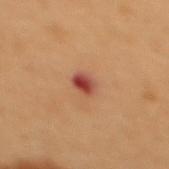  biopsy_status: not biopsied; imaged during a skin examination
  image:
    source: total-body photography crop
    field_of_view_mm: 15
  site: upper back
  patient:
    sex: female
    age_approx: 50
  lighting: cross-polarized
  automated_metrics:
    peripheral_color_asymmetry: 2.5
  lesion_size:
    long_diameter_mm_approx: 2.5